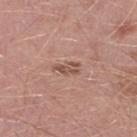Q: Is there a histopathology result?
A: catalogued during a skin exam; not biopsied
Q: What kind of image is this?
A: 15 mm crop, total-body photography
Q: What did automated image analysis measure?
A: a nevus-likeness score of about 5/100 and lesion-presence confidence of about 100/100
Q: How was the tile lit?
A: white-light
Q: Patient demographics?
A: male, aged around 50
Q: What is the anatomic site?
A: the right lower leg
Q: Lesion size?
A: ≈3 mm